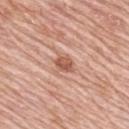No biopsy was performed on this lesion — it was imaged during a full skin examination and was not determined to be concerning.
Located on the upper back.
A roughly 15 mm field-of-view crop from a total-body skin photograph.
Longest diameter approximately 2.5 mm.
Imaged with white-light lighting.
The subject is a female aged 63 to 67.